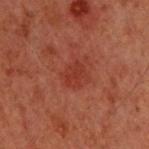Imaged during a routine full-body skin examination; the lesion was not biopsied and no histopathology is available. A male subject, approximately 60 years of age. A lesion tile, about 15 mm wide, cut from a 3D total-body photograph. Located on the upper back. An algorithmic analysis of the crop reported an area of roughly 4.5 mm², an eccentricity of roughly 0.6, and a symmetry-axis asymmetry near 0.25. And it measured an automated nevus-likeness rating near 0 out of 100. The lesion's longest dimension is about 2.5 mm.